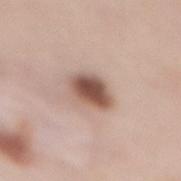Impression:
The lesion was photographed on a routine skin check and not biopsied; there is no pathology result.
Context:
The lesion's longest dimension is about 4 mm. Captured under white-light illumination. A region of skin cropped from a whole-body photographic capture, roughly 15 mm wide. A female subject aged approximately 50. An algorithmic analysis of the crop reported a mean CIELAB color near L≈53 a*≈19 b*≈25 and a normalized lesion–skin contrast near 11. And it measured a border-irregularity index near 1.5/10 and radial color variation of about 1.5. The software also gave a nevus-likeness score of about 100/100. The lesion is on the mid back.